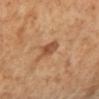<record>
  <biopsy_status>not biopsied; imaged during a skin examination</biopsy_status>
  <site>leg</site>
  <image>
    <source>total-body photography crop</source>
    <field_of_view_mm>15</field_of_view_mm>
  </image>
  <patient>
    <sex>female</sex>
    <age_approx>50</age_approx>
  </patient>
  <lighting>cross-polarized</lighting>
</record>